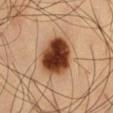Impression:
Imaged during a routine full-body skin examination; the lesion was not biopsied and no histopathology is available.
Image and clinical context:
On the abdomen. The patient is a male roughly 60 years of age. Cropped from a total-body skin-imaging series; the visible field is about 15 mm. The tile uses cross-polarized illumination. The lesion-visualizer software estimated a nevus-likeness score of about 100/100 and lesion-presence confidence of about 100/100.